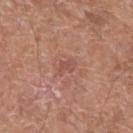biopsy status: no biopsy performed (imaged during a skin exam)
image: total-body-photography crop, ~15 mm field of view
lesion size: about 2.5 mm
tile lighting: white-light illumination
automated metrics: a mean CIELAB color near L≈51 a*≈24 b*≈27, roughly 7 lightness units darker than nearby skin, and a normalized border contrast of about 5; an automated nevus-likeness rating near 0 out of 100 and a detector confidence of about 100 out of 100 that the crop contains a lesion
site: the left lower leg
subject: male, aged 63 to 67